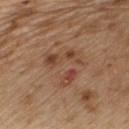Findings:
– follow-up — imaged on a skin check; not biopsied
– site — the upper back
– image source — total-body-photography crop, ~15 mm field of view
– lesion diameter — about 5.5 mm
– image-analysis metrics — an area of roughly 20 mm², an outline eccentricity of about 0.4 (0 = round, 1 = elongated), and two-axis asymmetry of about 0.3; roughly 6 lightness units darker than nearby skin and a lesion-to-skin contrast of about 5 (normalized; higher = more distinct); an automated nevus-likeness rating near 0 out of 100 and lesion-presence confidence of about 100/100
– lighting — white-light
– subject — male, approximately 70 years of age